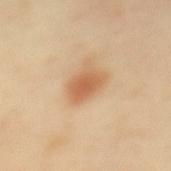workup = catalogued during a skin exam; not biopsied | anatomic site = the mid back | tile lighting = cross-polarized | imaging modality = total-body-photography crop, ~15 mm field of view | subject = female, aged approximately 40 | size = ~4 mm (longest diameter).